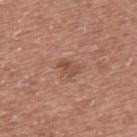This lesion was catalogued during total-body skin photography and was not selected for biopsy.
Cropped from a whole-body photographic skin survey; the tile spans about 15 mm.
The patient is a male about 60 years old.
Captured under white-light illumination.
On the upper back.